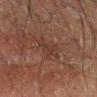Notes:
• image source · 15 mm crop, total-body photography
• site · the right lower leg
• illumination · cross-polarized illumination
• patient · male, aged approximately 50